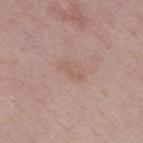From the upper back. Automated image analysis of the tile measured a border-irregularity index near 4.5/10, internal color variation of about 0 on a 0–10 scale, and radial color variation of about 0. The analysis additionally found a nevus-likeness score of about 0/100 and a detector confidence of about 100 out of 100 that the crop contains a lesion. This is a white-light tile. A female patient aged 48–52. A 15 mm close-up tile from a total-body photography series done for melanoma screening.